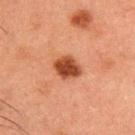Captured during whole-body skin photography for melanoma surveillance; the lesion was not biopsied. This image is a 15 mm lesion crop taken from a total-body photograph. A male patient, aged around 50. From the back.